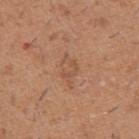{"biopsy_status": "not biopsied; imaged during a skin examination", "site": "left upper arm", "patient": {"sex": "male", "age_approx": 40}, "image": {"source": "total-body photography crop", "field_of_view_mm": 15}, "lesion_size": {"long_diameter_mm_approx": 3.5}, "lighting": "white-light"}